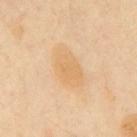automated_metrics:
  cielab_L: 70
  cielab_a: 17
  cielab_b: 41
  vs_skin_contrast_norm: 5.5
  color_variation_0_10: 2.5
  peripheral_color_asymmetry: 1.0
lighting: cross-polarized
site: back
lesion_size:
  long_diameter_mm_approx: 5.0
patient:
  sex: male
  age_approx: 50
image:
  source: total-body photography crop
  field_of_view_mm: 15